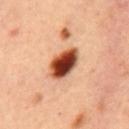* biopsy status — imaged on a skin check; not biopsied
* subject — female, approximately 45 years of age
* acquisition — 15 mm crop, total-body photography
* lesion diameter — ~4.5 mm (longest diameter)
* body site — the mid back